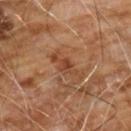Findings:
– follow-up · no biopsy performed (imaged during a skin exam)
– lighting · cross-polarized illumination
– lesion diameter · about 4 mm
– patient · male, approximately 60 years of age
– body site · the chest
– automated metrics · a footprint of about 5 mm², an outline eccentricity of about 0.9 (0 = round, 1 = elongated), and two-axis asymmetry of about 0.35
– acquisition · ~15 mm crop, total-body skin-cancer survey